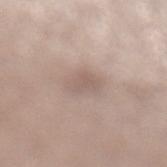| field | value |
|---|---|
| biopsy status | no biopsy performed (imaged during a skin exam) |
| patient | female, approximately 40 years of age |
| illumination | white-light illumination |
| anatomic site | the left lower leg |
| size | ≈3 mm |
| acquisition | ~15 mm tile from a whole-body skin photo |